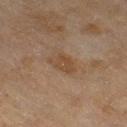The lesion was photographed on a routine skin check and not biopsied; there is no pathology result.
About 3 mm across.
The tile uses cross-polarized illumination.
A female subject, approximately 60 years of age.
The lesion is located on the right thigh.
Cropped from a total-body skin-imaging series; the visible field is about 15 mm.